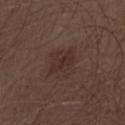The lesion was photographed on a routine skin check and not biopsied; there is no pathology result.
A male subject aged 68–72.
The tile uses white-light illumination.
From the front of the torso.
Cropped from a whole-body photographic skin survey; the tile spans about 15 mm.